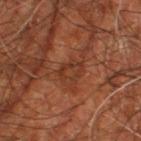A male subject in their 60s.
Automated image analysis of the tile measured a mean CIELAB color near L≈34 a*≈24 b*≈30, a lesion–skin lightness drop of about 6, and a normalized lesion–skin contrast near 5.5. It also reported a border-irregularity index near 2.5/10 and internal color variation of about 3 on a 0–10 scale. The software also gave a classifier nevus-likeness of about 0/100 and a detector confidence of about 90 out of 100 that the crop contains a lesion.
The lesion is located on the right leg.
Approximately 3 mm at its widest.
Cropped from a whole-body photographic skin survey; the tile spans about 15 mm.